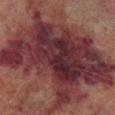| feature | finding |
|---|---|
| notes | catalogued during a skin exam; not biopsied |
| size | about 17 mm |
| TBP lesion metrics | a footprint of about 110 mm², an outline eccentricity of about 0.75 (0 = round, 1 = elongated), and a symmetry-axis asymmetry near 0.35; border irregularity of about 7.5 on a 0–10 scale and a peripheral color-asymmetry measure near 2 |
| subject | male, aged 73 to 77 |
| tile lighting | cross-polarized illumination |
| acquisition | ~15 mm crop, total-body skin-cancer survey |
| location | the right lower leg |